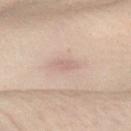{
  "biopsy_status": "not biopsied; imaged during a skin examination",
  "image": {
    "source": "total-body photography crop",
    "field_of_view_mm": 15
  },
  "lesion_size": {
    "long_diameter_mm_approx": 2.5
  },
  "automated_metrics": {
    "area_mm2_approx": 3.5,
    "shape_asymmetry": 0.3,
    "cielab_L": 51,
    "cielab_a": 14,
    "cielab_b": 20,
    "vs_skin_darker_L": 6.0,
    "vs_skin_contrast_norm": 4.5,
    "border_irregularity_0_10": 2.5,
    "color_variation_0_10": 0.5,
    "nevus_likeness_0_100": 0,
    "lesion_detection_confidence_0_100": 95
  },
  "lighting": "cross-polarized",
  "patient": {
    "sex": "female",
    "age_approx": 30
  },
  "site": "left forearm"
}